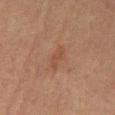Q: Was a biopsy performed?
A: total-body-photography surveillance lesion; no biopsy
Q: What is the anatomic site?
A: the mid back
Q: How was this image acquired?
A: 15 mm crop, total-body photography
Q: Automated lesion metrics?
A: a footprint of about 4 mm², an outline eccentricity of about 0.9 (0 = round, 1 = elongated), and a shape-asymmetry score of about 0.4 (0 = symmetric); a lesion color around L≈38 a*≈18 b*≈25 in CIELAB and a lesion–skin lightness drop of about 5; a classifier nevus-likeness of about 0/100 and a detector confidence of about 100 out of 100 that the crop contains a lesion
Q: What are the patient's age and sex?
A: male, aged around 65
Q: How was the tile lit?
A: cross-polarized